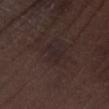biopsy status: total-body-photography surveillance lesion; no biopsy | acquisition: 15 mm crop, total-body photography | patient: male, in their 70s | body site: the left lower leg.